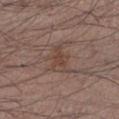| field | value |
|---|---|
| notes | total-body-photography surveillance lesion; no biopsy |
| body site | the left lower leg |
| illumination | white-light |
| imaging modality | ~15 mm crop, total-body skin-cancer survey |
| TBP lesion metrics | border irregularity of about 3.5 on a 0–10 scale, a within-lesion color-variation index near 2.5/10, and radial color variation of about 1; a classifier nevus-likeness of about 0/100 and a detector confidence of about 100 out of 100 that the crop contains a lesion |
| subject | male, about 40 years old |